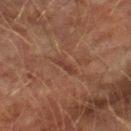Impression:
Part of a total-body skin-imaging series; this lesion was reviewed on a skin check and was not flagged for biopsy.
Acquisition and patient details:
The lesion is located on the right lower leg. A male subject, aged 73–77. A 15 mm close-up extracted from a 3D total-body photography capture.